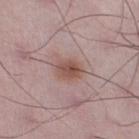Notes:
- biopsy status · imaged on a skin check; not biopsied
- lighting · white-light
- patient · male, in their 50s
- location · the right lower leg
- imaging modality · 15 mm crop, total-body photography
- image-analysis metrics · a footprint of about 6.5 mm², an outline eccentricity of about 0.65 (0 = round, 1 = elongated), and a shape-asymmetry score of about 0.2 (0 = symmetric); an average lesion color of about L≈51 a*≈20 b*≈24 (CIELAB), roughly 10 lightness units darker than nearby skin, and a normalized lesion–skin contrast near 8; a classifier nevus-likeness of about 90/100 and lesion-presence confidence of about 100/100